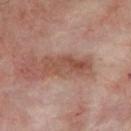Notes:
• subject: male, aged around 50
• lesion diameter: ≈6 mm
• lighting: cross-polarized illumination
• anatomic site: the upper back
• acquisition: total-body-photography crop, ~15 mm field of view